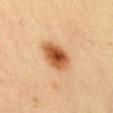Findings:
- biopsy status · no biopsy performed (imaged during a skin exam)
- location · the mid back
- lighting · cross-polarized
- subject · female, aged around 40
- imaging modality · ~15 mm tile from a whole-body skin photo